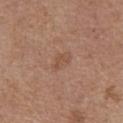<case>
  <biopsy_status>not biopsied; imaged during a skin examination</biopsy_status>
  <site>abdomen</site>
  <automated_metrics>
    <nevus_likeness_0_100>0</nevus_likeness_0_100>
    <lesion_detection_confidence_0_100>100</lesion_detection_confidence_0_100>
  </automated_metrics>
  <image>
    <source>total-body photography crop</source>
    <field_of_view_mm>15</field_of_view_mm>
  </image>
  <patient>
    <sex>female</sex>
    <age_approx>65</age_approx>
  </patient>
  <lighting>white-light</lighting>
</case>